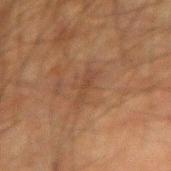This is a cross-polarized tile. On the right forearm. A male patient, aged around 80. Cropped from a whole-body photographic skin survey; the tile spans about 15 mm. Automated image analysis of the tile measured an area of roughly 4 mm² and a symmetry-axis asymmetry near 0.45. The software also gave a lesion color around L≈34 a*≈16 b*≈25 in CIELAB, roughly 5 lightness units darker than nearby skin, and a lesion-to-skin contrast of about 5 (normalized; higher = more distinct). The analysis additionally found a border-irregularity index near 7/10 and radial color variation of about 0. The analysis additionally found a detector confidence of about 85 out of 100 that the crop contains a lesion. The recorded lesion diameter is about 3.5 mm.